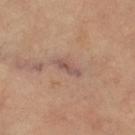Located on the leg. The patient is a female aged 68–72. About 3 mm across. The tile uses cross-polarized illumination. A region of skin cropped from a whole-body photographic capture, roughly 15 mm wide.